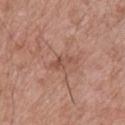{
  "biopsy_status": "not biopsied; imaged during a skin examination",
  "site": "upper back",
  "image": {
    "source": "total-body photography crop",
    "field_of_view_mm": 15
  },
  "lesion_size": {
    "long_diameter_mm_approx": 3.5
  },
  "automated_metrics": {
    "area_mm2_approx": 4.0,
    "eccentricity": 0.9,
    "shape_asymmetry": 0.45,
    "border_irregularity_0_10": 5.0,
    "peripheral_color_asymmetry": 0.5,
    "lesion_detection_confidence_0_100": 100
  },
  "lighting": "white-light",
  "patient": {
    "sex": "male",
    "age_approx": 65
  }
}